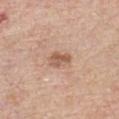Notes:
• site — the front of the torso
• acquisition — ~15 mm tile from a whole-body skin photo
• lighting — white-light
• patient — male, aged 58 to 62